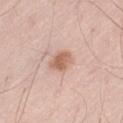Q: Was this lesion biopsied?
A: total-body-photography surveillance lesion; no biopsy
Q: What is the imaging modality?
A: total-body-photography crop, ~15 mm field of view
Q: What did automated image analysis measure?
A: a lesion-detection confidence of about 100/100
Q: Where on the body is the lesion?
A: the left thigh
Q: How large is the lesion?
A: ~2.5 mm (longest diameter)
Q: How was the tile lit?
A: white-light
Q: Patient demographics?
A: male, in their mid-50s